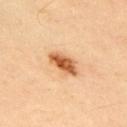Part of a total-body skin-imaging series; this lesion was reviewed on a skin check and was not flagged for biopsy.
A 15 mm close-up extracted from a 3D total-body photography capture.
The lesion is on the upper back.
Longest diameter approximately 4 mm.
Automated tile analysis of the lesion measured roughly 17 lightness units darker than nearby skin. The analysis additionally found a border-irregularity index near 2.5/10, a color-variation rating of about 4/10, and radial color variation of about 1.5.
A male subject, in their 50s.